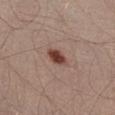An algorithmic analysis of the crop reported a classifier nevus-likeness of about 100/100 and a detector confidence of about 100 out of 100 that the crop contains a lesion. A male patient aged around 55. The lesion is on the abdomen. Cropped from a whole-body photographic skin survey; the tile spans about 15 mm.